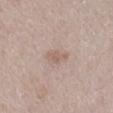The lesion was photographed on a routine skin check and not biopsied; there is no pathology result.
Captured under white-light illumination.
Approximately 3 mm at its widest.
The patient is a female aged 28 to 32.
The lesion is located on the mid back.
Automated tile analysis of the lesion measured a border-irregularity rating of about 3/10 and peripheral color asymmetry of about 0.5.
A region of skin cropped from a whole-body photographic capture, roughly 15 mm wide.